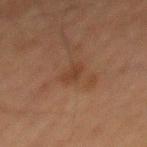biopsy status=total-body-photography surveillance lesion; no biopsy | lesion diameter=about 3 mm | illumination=cross-polarized illumination | automated metrics=a footprint of about 3.5 mm², a shape eccentricity near 0.85, and a symmetry-axis asymmetry near 0.25 | patient=male, roughly 65 years of age | acquisition=~15 mm crop, total-body skin-cancer survey | anatomic site=the back.